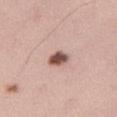biopsy_status: not biopsied; imaged during a skin examination
site: right thigh
image:
  source: total-body photography crop
  field_of_view_mm: 15
lighting: white-light
patient:
  sex: female
  age_approx: 35